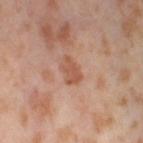Q: Is there a histopathology result?
A: total-body-photography surveillance lesion; no biopsy
Q: What are the patient's age and sex?
A: female, about 55 years old
Q: Illumination type?
A: cross-polarized illumination
Q: How was this image acquired?
A: 15 mm crop, total-body photography
Q: What did automated image analysis measure?
A: a footprint of about 4.5 mm², a shape eccentricity near 0.8, and a symmetry-axis asymmetry near 0.3; an average lesion color of about L≈55 a*≈24 b*≈32 (CIELAB), about 9 CIELAB-L* units darker than the surrounding skin, and a normalized lesion–skin contrast near 6.5; a nevus-likeness score of about 0/100
Q: What is the anatomic site?
A: the left thigh
Q: Lesion size?
A: ≈3 mm